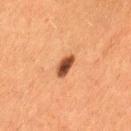<record>
<biopsy_status>not biopsied; imaged during a skin examination</biopsy_status>
<patient>
  <sex>female</sex>
  <age_approx>50</age_approx>
</patient>
<automated_metrics>
  <cielab_L>41</cielab_L>
  <cielab_a>24</cielab_a>
  <cielab_b>32</cielab_b>
  <vs_skin_darker_L>16.0</vs_skin_darker_L>
  <vs_skin_contrast_norm>12.5</vs_skin_contrast_norm>
  <nevus_likeness_0_100>100</nevus_likeness_0_100>
  <lesion_detection_confidence_0_100>100</lesion_detection_confidence_0_100>
</automated_metrics>
<image>
  <source>total-body photography crop</source>
  <field_of_view_mm>15</field_of_view_mm>
</image>
<site>left thigh</site>
<lesion_size>
  <long_diameter_mm_approx>3.0</long_diameter_mm_approx>
</lesion_size>
</record>